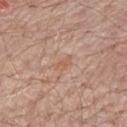Q: Was this lesion biopsied?
A: imaged on a skin check; not biopsied
Q: What did automated image analysis measure?
A: a lesion area of about 3 mm², an eccentricity of roughly 0.85, and a symmetry-axis asymmetry near 0.4; an average lesion color of about L≈59 a*≈20 b*≈30 (CIELAB), roughly 6 lightness units darker than nearby skin, and a normalized lesion–skin contrast near 5
Q: How was the tile lit?
A: white-light illumination
Q: Where on the body is the lesion?
A: the mid back
Q: Who is the patient?
A: male, approximately 70 years of age
Q: What is the imaging modality?
A: 15 mm crop, total-body photography